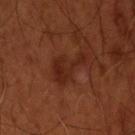This lesion was catalogued during total-body skin photography and was not selected for biopsy. The lesion-visualizer software estimated an average lesion color of about L≈25 a*≈23 b*≈27 (CIELAB) and about 6 CIELAB-L* units darker than the surrounding skin. The software also gave a border-irregularity index near 7/10, internal color variation of about 2 on a 0–10 scale, and radial color variation of about 0.5. From the head or neck. Cropped from a whole-body photographic skin survey; the tile spans about 15 mm. A male patient, aged 53 to 57. Approximately 4.5 mm at its widest. Imaged with cross-polarized lighting.